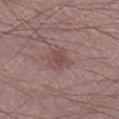lesion size: ≈2.5 mm
location: the right lower leg
patient: male, aged 33–37
illumination: white-light illumination
automated lesion analysis: an area of roughly 5 mm² and a shape-asymmetry score of about 0.35 (0 = symmetric); a border-irregularity index near 3.5/10, a color-variation rating of about 1.5/10, and peripheral color asymmetry of about 0.5; a nevus-likeness score of about 0/100 and a detector confidence of about 100 out of 100 that the crop contains a lesion
image source: ~15 mm crop, total-body skin-cancer survey